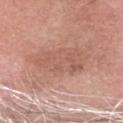size: ~7.5 mm (longest diameter)
lighting: white-light
acquisition: 15 mm crop, total-body photography
patient: male, roughly 70 years of age
site: the head or neck